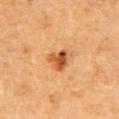Imaged during a routine full-body skin examination; the lesion was not biopsied and no histopathology is available.
A 15 mm close-up tile from a total-body photography series done for melanoma screening.
The lesion is on the chest.
A male subject in their mid- to late 80s or older.